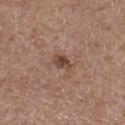Impression: This lesion was catalogued during total-body skin photography and was not selected for biopsy. Clinical summary: Longest diameter approximately 2.5 mm. A close-up tile cropped from a whole-body skin photograph, about 15 mm across. The patient is a female aged approximately 65. Located on the right thigh.